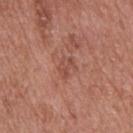Assessment:
No biopsy was performed on this lesion — it was imaged during a full skin examination and was not determined to be concerning.
Context:
On the upper back. The subject is a male about 70 years old. Captured under white-light illumination. The total-body-photography lesion software estimated a lesion area of about 3.5 mm², a shape eccentricity near 0.8, and a shape-asymmetry score of about 0.4 (0 = symmetric). And it measured a border-irregularity rating of about 4/10 and radial color variation of about 0.5. A close-up tile cropped from a whole-body skin photograph, about 15 mm across.